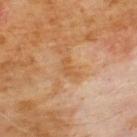Context:
A 15 mm close-up tile from a total-body photography series done for melanoma screening. The patient is a male aged approximately 60. The total-body-photography lesion software estimated a lesion area of about 2.5 mm², an outline eccentricity of about 0.85 (0 = round, 1 = elongated), and a symmetry-axis asymmetry near 0.5. And it measured a border-irregularity rating of about 5.5/10 and radial color variation of about 0. The lesion is located on the front of the torso. About 2.5 mm across.